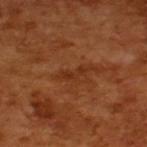Case summary:
• biopsy status · total-body-photography surveillance lesion; no biopsy
• patient · male, in their mid-60s
• acquisition · ~15 mm crop, total-body skin-cancer survey